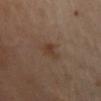Part of a total-body skin-imaging series; this lesion was reviewed on a skin check and was not flagged for biopsy. Captured under cross-polarized illumination. Automated image analysis of the tile measured a footprint of about 3.5 mm². The software also gave a mean CIELAB color near L≈37 a*≈16 b*≈27 and a normalized border contrast of about 6.5. And it measured lesion-presence confidence of about 100/100. A female subject aged 58–62. Measured at roughly 2.5 mm in maximum diameter. On the front of the torso. A lesion tile, about 15 mm wide, cut from a 3D total-body photograph.